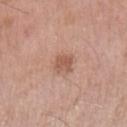biopsy status: imaged on a skin check; not biopsied | tile lighting: white-light illumination | patient: male, aged 53 to 57 | image: ~15 mm crop, total-body skin-cancer survey | size: ~2.5 mm (longest diameter) | location: the left upper arm.